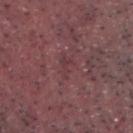notes — no biopsy performed (imaged during a skin exam)
size — ~2.5 mm (longest diameter)
site — the chest
acquisition — ~15 mm tile from a whole-body skin photo
tile lighting — white-light
automated lesion analysis — about 5 CIELAB-L* units darker than the surrounding skin; lesion-presence confidence of about 75/100
subject — male, roughly 40 years of age